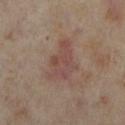Assessment: No biopsy was performed on this lesion — it was imaged during a full skin examination and was not determined to be concerning. Background: The lesion is located on the left lower leg. A 15 mm close-up extracted from a 3D total-body photography capture. About 6 mm across. A female subject approximately 35 years of age.